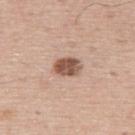follow-up: total-body-photography surveillance lesion; no biopsy | subject: male, in their mid-60s | lesion diameter: ~3 mm (longest diameter) | tile lighting: white-light | acquisition: ~15 mm crop, total-body skin-cancer survey | site: the upper back.